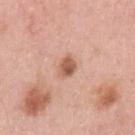No biopsy was performed on this lesion — it was imaged during a full skin examination and was not determined to be concerning. A female subject, aged 38 to 42. Approximately 2.5 mm at its widest. Automated image analysis of the tile measured an average lesion color of about L≈58 a*≈23 b*≈31 (CIELAB), a lesion–skin lightness drop of about 13, and a lesion-to-skin contrast of about 9 (normalized; higher = more distinct). The software also gave a border-irregularity index near 2/10. It also reported an automated nevus-likeness rating near 85 out of 100 and lesion-presence confidence of about 100/100. From the left upper arm. Cropped from a whole-body photographic skin survey; the tile spans about 15 mm.